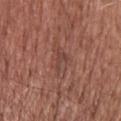Q: Was a biopsy performed?
A: no biopsy performed (imaged during a skin exam)
Q: What did automated image analysis measure?
A: a classifier nevus-likeness of about 0/100 and lesion-presence confidence of about 90/100
Q: What are the patient's age and sex?
A: male, about 55 years old
Q: Lesion size?
A: ~3 mm (longest diameter)
Q: What kind of image is this?
A: ~15 mm tile from a whole-body skin photo
Q: What is the anatomic site?
A: the upper back
Q: What lighting was used for the tile?
A: white-light